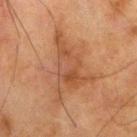Notes:
* biopsy status — no biopsy performed (imaged during a skin exam)
* acquisition — ~15 mm crop, total-body skin-cancer survey
* patient — male, aged around 80
* diameter — ≈8 mm
* lighting — cross-polarized
* site — the right thigh